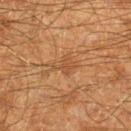This lesion was catalogued during total-body skin photography and was not selected for biopsy.
A male subject in their 60s.
The lesion's longest dimension is about 3 mm.
On the right lower leg.
A region of skin cropped from a whole-body photographic capture, roughly 15 mm wide.